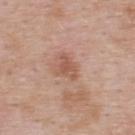Q: Patient demographics?
A: male, about 55 years old
Q: How large is the lesion?
A: about 3 mm
Q: What did automated image analysis measure?
A: a footprint of about 5 mm² and a shape-asymmetry score of about 0.45 (0 = symmetric); roughly 9 lightness units darker than nearby skin and a normalized border contrast of about 6; a nevus-likeness score of about 0/100 and a lesion-detection confidence of about 100/100
Q: What is the anatomic site?
A: the upper back
Q: How was the tile lit?
A: white-light
Q: What kind of image is this?
A: ~15 mm crop, total-body skin-cancer survey